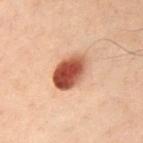Imaged during a routine full-body skin examination; the lesion was not biopsied and no histopathology is available. Imaged with cross-polarized lighting. A 15 mm crop from a total-body photograph taken for skin-cancer surveillance. The subject is a male approximately 40 years of age. On the front of the torso. Automated image analysis of the tile measured a border-irregularity rating of about 1/10, internal color variation of about 8.5 on a 0–10 scale, and a peripheral color-asymmetry measure near 3. Measured at roughly 4.5 mm in maximum diameter.